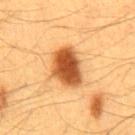Captured during whole-body skin photography for melanoma surveillance; the lesion was not biopsied. A male subject, about 60 years old. A roughly 15 mm field-of-view crop from a total-body skin photograph. The lesion is on the abdomen.